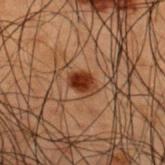No biopsy was performed on this lesion — it was imaged during a full skin examination and was not determined to be concerning. A region of skin cropped from a whole-body photographic capture, roughly 15 mm wide. The lesion-visualizer software estimated a lesion area of about 5 mm², a shape eccentricity near 0.75, and a symmetry-axis asymmetry near 0.25. It also reported a classifier nevus-likeness of about 100/100 and a detector confidence of about 100 out of 100 that the crop contains a lesion. A male patient, aged 48–52. From the back. The tile uses cross-polarized illumination.